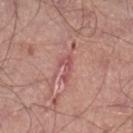follow-up = total-body-photography surveillance lesion; no biopsy | anatomic site = the right lower leg | image = ~15 mm crop, total-body skin-cancer survey | size = about 3 mm | tile lighting = white-light illumination | image-analysis metrics = an average lesion color of about L≈53 a*≈28 b*≈23 (CIELAB), a lesion–skin lightness drop of about 8, and a normalized lesion–skin contrast near 6; an automated nevus-likeness rating near 0 out of 100 and a detector confidence of about 65 out of 100 that the crop contains a lesion | patient = male, aged 38–42.